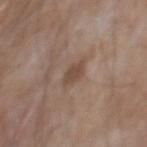Assessment:
Recorded during total-body skin imaging; not selected for excision or biopsy.
Context:
A 15 mm close-up extracted from a 3D total-body photography capture. Captured under white-light illumination. The lesion's longest dimension is about 3 mm. The patient is a male aged 58–62. The lesion is located on the mid back.